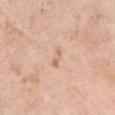{
  "biopsy_status": "not biopsied; imaged during a skin examination",
  "site": "left upper arm",
  "automated_metrics": {
    "area_mm2_approx": 2.5,
    "eccentricity": 0.95,
    "shape_asymmetry": 0.35,
    "nevus_likeness_0_100": 0,
    "lesion_detection_confidence_0_100": 100
  },
  "patient": {
    "sex": "male",
    "age_approx": 60
  },
  "image": {
    "source": "total-body photography crop",
    "field_of_view_mm": 15
  },
  "lighting": "white-light"
}